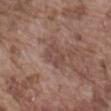Captured during whole-body skin photography for melanoma surveillance; the lesion was not biopsied.
A male patient, about 75 years old.
Located on the abdomen.
A 15 mm close-up tile from a total-body photography series done for melanoma screening.